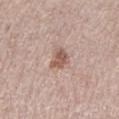workup = catalogued during a skin exam; not biopsied